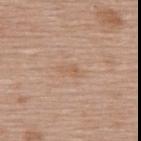biopsy status = imaged on a skin check; not biopsied
subject = female, aged approximately 60
location = the upper back
size = about 2.5 mm
imaging modality = 15 mm crop, total-body photography